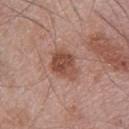Impression:
This lesion was catalogued during total-body skin photography and was not selected for biopsy.
Clinical summary:
A 15 mm crop from a total-body photograph taken for skin-cancer surveillance. This is a white-light tile. Approximately 4 mm at its widest. A male subject aged around 75. From the chest.